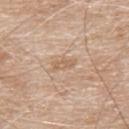  biopsy_status: not biopsied; imaged during a skin examination
  patient:
    sex: male
    age_approx: 80
  lesion_size:
    long_diameter_mm_approx: 2.5
  image:
    source: total-body photography crop
    field_of_view_mm: 15
  lighting: white-light
  site: upper back